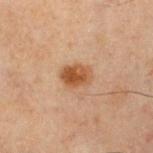Assessment:
This lesion was catalogued during total-body skin photography and was not selected for biopsy.
Image and clinical context:
A 15 mm crop from a total-body photograph taken for skin-cancer surveillance. The total-body-photography lesion software estimated an automated nevus-likeness rating near 95 out of 100. A male subject aged 58–62. The lesion is on the front of the torso. The tile uses cross-polarized illumination.